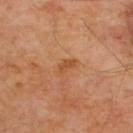* size: ≈2.5 mm
* subject: male, aged around 65
* imaging modality: ~15 mm tile from a whole-body skin photo
* body site: the upper back
* tile lighting: cross-polarized illumination